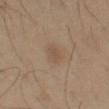The lesion was photographed on a routine skin check and not biopsied; there is no pathology result.
On the left thigh.
The lesion-visualizer software estimated a footprint of about 4.5 mm², an outline eccentricity of about 0.65 (0 = round, 1 = elongated), and a symmetry-axis asymmetry near 0.25. And it measured a nevus-likeness score of about 10/100 and a lesion-detection confidence of about 100/100.
A close-up tile cropped from a whole-body skin photograph, about 15 mm across.
A male subject, approximately 55 years of age.
The tile uses cross-polarized illumination.